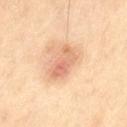The lesion was tiled from a total-body skin photograph and was not biopsied. On the left thigh. The lesion-visualizer software estimated a footprint of about 7.5 mm², an eccentricity of roughly 0.85, and a shape-asymmetry score of about 0.25 (0 = symmetric). And it measured a border-irregularity index near 3/10, internal color variation of about 7 on a 0–10 scale, and radial color variation of about 2.5. Measured at roughly 4 mm in maximum diameter. A male subject in their mid- to late 60s. The tile uses cross-polarized illumination. A lesion tile, about 15 mm wide, cut from a 3D total-body photograph.